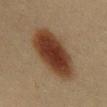{
  "biopsy_status": "not biopsied; imaged during a skin examination",
  "lesion_size": {
    "long_diameter_mm_approx": 8.0
  },
  "image": {
    "source": "total-body photography crop",
    "field_of_view_mm": 15
  },
  "lighting": "cross-polarized",
  "site": "mid back",
  "patient": {
    "sex": "female",
    "age_approx": 30
  }
}